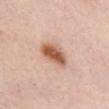This lesion was catalogued during total-body skin photography and was not selected for biopsy. This image is a 15 mm lesion crop taken from a total-body photograph. Longest diameter approximately 4 mm. Captured under white-light illumination. A female patient, aged 48 to 52. Located on the front of the torso. Automated tile analysis of the lesion measured a footprint of about 9 mm² and an outline eccentricity of about 0.8 (0 = round, 1 = elongated). The software also gave a classifier nevus-likeness of about 100/100 and a lesion-detection confidence of about 100/100.